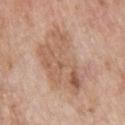Captured under white-light illumination.
The lesion is located on the back.
A male subject, aged around 60.
A 15 mm close-up tile from a total-body photography series done for melanoma screening.
Approximately 8.5 mm at its widest.